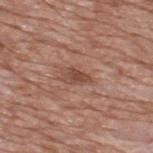illumination: white-light | subject: male, aged 58 to 62 | automated metrics: an area of roughly 4 mm² and a symmetry-axis asymmetry near 0.3; a mean CIELAB color near L≈47 a*≈22 b*≈28 and a normalized border contrast of about 7; a within-lesion color-variation index near 2.5/10 and radial color variation of about 0.5; an automated nevus-likeness rating near 25 out of 100 and a lesion-detection confidence of about 100/100 | location: the upper back | image source: ~15 mm tile from a whole-body skin photo | lesion size: ~3 mm (longest diameter).